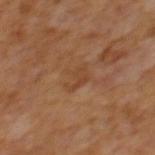No biopsy was performed on this lesion — it was imaged during a full skin examination and was not determined to be concerning.
A male subject aged 63–67.
A 15 mm crop from a total-body photograph taken for skin-cancer surveillance.
The lesion-visualizer software estimated about 5 CIELAB-L* units darker than the surrounding skin and a normalized border contrast of about 5. The analysis additionally found a border-irregularity index near 5.5/10, a color-variation rating of about 1/10, and radial color variation of about 0.5.
Approximately 3 mm at its widest.
The lesion is on the mid back.
Imaged with cross-polarized lighting.